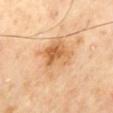The lesion was photographed on a routine skin check and not biopsied; there is no pathology result. The total-body-photography lesion software estimated an eccentricity of roughly 0.6 and a shape-asymmetry score of about 0.2 (0 = symmetric). And it measured an average lesion color of about L≈67 a*≈21 b*≈41 (CIELAB), a lesion–skin lightness drop of about 10, and a normalized border contrast of about 7. And it measured a border-irregularity rating of about 3/10 and a peripheral color-asymmetry measure near 2.5. A male patient, in their mid-60s. From the mid back. A region of skin cropped from a whole-body photographic capture, roughly 15 mm wide. Captured under cross-polarized illumination.